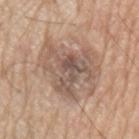Captured during whole-body skin photography for melanoma surveillance; the lesion was not biopsied. A close-up tile cropped from a whole-body skin photograph, about 15 mm across. Automated tile analysis of the lesion measured a mean CIELAB color near L≈55 a*≈16 b*≈25, about 10 CIELAB-L* units darker than the surrounding skin, and a normalized lesion–skin contrast near 7.5. And it measured border irregularity of about 7 on a 0–10 scale. And it measured a classifier nevus-likeness of about 10/100. The lesion is located on the left upper arm. The subject is a male aged around 70. Imaged with white-light lighting.